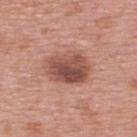From the back. This is a white-light tile. Measured at roughly 5 mm in maximum diameter. A female subject aged around 40. A roughly 15 mm field-of-view crop from a total-body skin photograph.